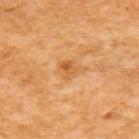| key | value |
|---|---|
| biopsy status | total-body-photography surveillance lesion; no biopsy |
| lighting | cross-polarized |
| acquisition | 15 mm crop, total-body photography |
| TBP lesion metrics | border irregularity of about 3 on a 0–10 scale, a color-variation rating of about 5.5/10, and peripheral color asymmetry of about 2; a nevus-likeness score of about 10/100 and a detector confidence of about 100 out of 100 that the crop contains a lesion |
| size | about 3 mm |
| subject | female, aged 53–57 |
| site | the upper back |